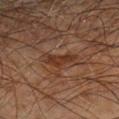follow-up=catalogued during a skin exam; not biopsied | tile lighting=cross-polarized illumination | anatomic site=the left forearm | image source=total-body-photography crop, ~15 mm field of view | size=~3.5 mm (longest diameter) | subject=male, aged 68–72.